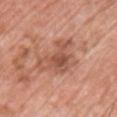No biopsy was performed on this lesion — it was imaged during a full skin examination and was not determined to be concerning. The tile uses white-light illumination. A lesion tile, about 15 mm wide, cut from a 3D total-body photograph. The patient is a male about 60 years old. The lesion is located on the chest. About 6 mm across.